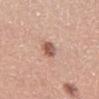{"biopsy_status": "not biopsied; imaged during a skin examination", "site": "right lower leg", "patient": {"sex": "male", "age_approx": 35}, "automated_metrics": {"border_irregularity_0_10": 2.0, "color_variation_0_10": 4.5, "peripheral_color_asymmetry": 1.5}, "image": {"source": "total-body photography crop", "field_of_view_mm": 15}}